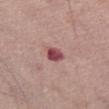biopsy_status: not biopsied; imaged during a skin examination
site: left thigh
image:
  source: total-body photography crop
  field_of_view_mm: 15
patient:
  sex: male
  age_approx: 70